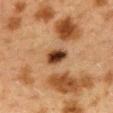{"biopsy_status": "not biopsied; imaged during a skin examination", "lighting": "cross-polarized", "site": "back", "image": {"source": "total-body photography crop", "field_of_view_mm": 15}, "lesion_size": {"long_diameter_mm_approx": 3.0}, "patient": {"sex": "female", "age_approx": 40}}